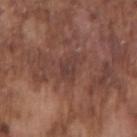workup: no biopsy performed (imaged during a skin exam) | acquisition: 15 mm crop, total-body photography | location: the right upper arm | automated metrics: an area of roughly 4 mm²; an automated nevus-likeness rating near 0 out of 100 and a lesion-detection confidence of about 95/100 | illumination: white-light | subject: male, in their mid-70s.